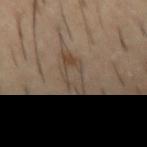Clinical impression:
No biopsy was performed on this lesion — it was imaged during a full skin examination and was not determined to be concerning.
Clinical summary:
This image is a 15 mm lesion crop taken from a total-body photograph. Captured under cross-polarized illumination. The recorded lesion diameter is about 5 mm. A male subject aged approximately 55. An algorithmic analysis of the crop reported a lesion area of about 7.5 mm². It also reported a lesion color around L≈43 a*≈11 b*≈24 in CIELAB and a normalized lesion–skin contrast near 5.5. It also reported border irregularity of about 4.5 on a 0–10 scale, internal color variation of about 6 on a 0–10 scale, and radial color variation of about 1.5. Located on the arm.